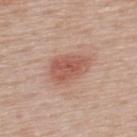{
  "image": {
    "source": "total-body photography crop",
    "field_of_view_mm": 15
  },
  "lesion_size": {
    "long_diameter_mm_approx": 4.5
  },
  "patient": {
    "sex": "male",
    "age_approx": 60
  },
  "site": "upper back"
}